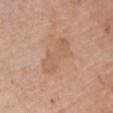Q: Is there a histopathology result?
A: catalogued during a skin exam; not biopsied
Q: Lesion location?
A: the front of the torso
Q: How large is the lesion?
A: ≈6 mm
Q: Patient demographics?
A: female, aged approximately 75
Q: Automated lesion metrics?
A: an area of roughly 11 mm² and a shape-asymmetry score of about 0.3 (0 = symmetric); a mean CIELAB color near L≈59 a*≈20 b*≈33, roughly 6 lightness units darker than nearby skin, and a lesion-to-skin contrast of about 5 (normalized; higher = more distinct); an automated nevus-likeness rating near 0 out of 100 and a lesion-detection confidence of about 100/100
Q: What is the imaging modality?
A: total-body-photography crop, ~15 mm field of view